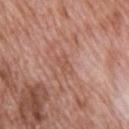biopsy_status: not biopsied; imaged during a skin examination
lesion_size:
  long_diameter_mm_approx: 2.5
patient:
  sex: male
  age_approx: 70
image:
  source: total-body photography crop
  field_of_view_mm: 15
lighting: white-light
site: upper back